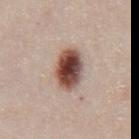Imaged during a routine full-body skin examination; the lesion was not biopsied and no histopathology is available. Longest diameter approximately 5 mm. Located on the chest. A 15 mm close-up tile from a total-body photography series done for melanoma screening. Automated tile analysis of the lesion measured an area of roughly 12 mm², a shape eccentricity near 0.75, and a symmetry-axis asymmetry near 0.15. The software also gave border irregularity of about 1.5 on a 0–10 scale, a within-lesion color-variation index near 8.5/10, and radial color variation of about 2.5. And it measured a nevus-likeness score of about 100/100 and a lesion-detection confidence of about 100/100. Imaged with white-light lighting. A male subject, about 45 years old.